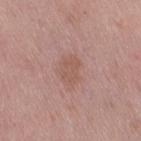{"biopsy_status": "not biopsied; imaged during a skin examination", "patient": {"sex": "female", "age_approx": 65}, "lighting": "white-light", "image": {"source": "total-body photography crop", "field_of_view_mm": 15}, "site": "right thigh"}